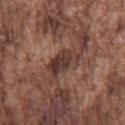site: the chest | patient: male, aged 73 to 77 | image source: 15 mm crop, total-body photography | diameter: ≈4.5 mm.